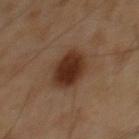Findings:
* follow-up: catalogued during a skin exam; not biopsied
* automated metrics: an area of roughly 13 mm² and an outline eccentricity of about 0.5 (0 = round, 1 = elongated); a border-irregularity index near 1.5/10, a color-variation rating of about 3/10, and radial color variation of about 1
* imaging modality: total-body-photography crop, ~15 mm field of view
* body site: the chest
* subject: male, about 60 years old